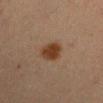{"biopsy_status": "not biopsied; imaged during a skin examination", "patient": {"sex": "male", "age_approx": 60}, "site": "right upper arm", "lighting": "cross-polarized", "automated_metrics": {"vs_skin_darker_L": 9.0, "nevus_likeness_0_100": 100, "lesion_detection_confidence_0_100": 100}, "lesion_size": {"long_diameter_mm_approx": 3.5}, "image": {"source": "total-body photography crop", "field_of_view_mm": 15}}